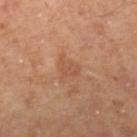Imaged during a routine full-body skin examination; the lesion was not biopsied and no histopathology is available. A male patient, aged approximately 50. The lesion is located on the leg. A region of skin cropped from a whole-body photographic capture, roughly 15 mm wide. Automated image analysis of the tile measured a footprint of about 4.5 mm².